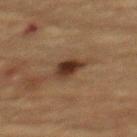  biopsy_status: not biopsied; imaged during a skin examination
  lesion_size:
    long_diameter_mm_approx: 3.5
  site: upper back
  patient:
    sex: female
    age_approx: 70
  image:
    source: total-body photography crop
    field_of_view_mm: 15
  lighting: cross-polarized